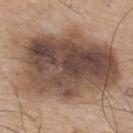The lesion was photographed on a routine skin check and not biopsied; there is no pathology result.